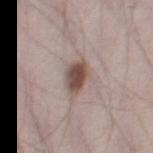<case>
<biopsy_status>not biopsied; imaged during a skin examination</biopsy_status>
<image>
  <source>total-body photography crop</source>
  <field_of_view_mm>15</field_of_view_mm>
</image>
<automated_metrics>
  <eccentricity>0.75</eccentricity>
  <shape_asymmetry>0.2</shape_asymmetry>
  <border_irregularity_0_10>2.0</border_irregularity_0_10>
  <peripheral_color_asymmetry>1.0</peripheral_color_asymmetry>
</automated_metrics>
<lesion_size>
  <long_diameter_mm_approx>4.0</long_diameter_mm_approx>
</lesion_size>
<patient>
  <sex>male</sex>
  <age_approx>70</age_approx>
</patient>
<site>right thigh</site>
</case>